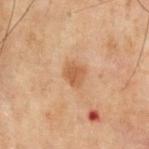notes: imaged on a skin check; not biopsied | patient: male, aged approximately 70 | automated metrics: a lesion color around L≈43 a*≈18 b*≈30 in CIELAB and a lesion-to-skin contrast of about 7 (normalized; higher = more distinct); a border-irregularity rating of about 2/10, internal color variation of about 1.5 on a 0–10 scale, and a peripheral color-asymmetry measure near 0.5 | site: the chest | lighting: cross-polarized | imaging modality: total-body-photography crop, ~15 mm field of view | lesion size: about 2.5 mm.